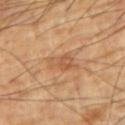Case summary:
* workup · no biopsy performed (imaged during a skin exam)
* automated metrics · two-axis asymmetry of about 0.35; a mean CIELAB color near L≈54 a*≈21 b*≈36 and a lesion-to-skin contrast of about 5.5 (normalized; higher = more distinct); a border-irregularity rating of about 4.5/10, a color-variation rating of about 2.5/10, and a peripheral color-asymmetry measure near 1
* acquisition · ~15 mm tile from a whole-body skin photo
* anatomic site · the left upper arm
* size · ≈3.5 mm
* illumination · cross-polarized
* subject · male, in their 60s